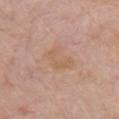Part of a total-body skin-imaging series; this lesion was reviewed on a skin check and was not flagged for biopsy.
Approximately 4 mm at its widest.
Automated image analysis of the tile measured a border-irregularity rating of about 3/10, a color-variation rating of about 2/10, and a peripheral color-asymmetry measure near 0.5.
A male patient aged around 80.
The lesion is located on the chest.
A 15 mm close-up tile from a total-body photography series done for melanoma screening.